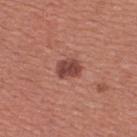Assessment:
Imaged during a routine full-body skin examination; the lesion was not biopsied and no histopathology is available.
Background:
This is a white-light tile. Longest diameter approximately 3 mm. On the back. This image is a 15 mm lesion crop taken from a total-body photograph. A female patient, aged around 50.